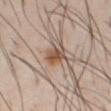{
  "image": {
    "source": "total-body photography crop",
    "field_of_view_mm": 15
  },
  "site": "abdomen",
  "patient": {
    "sex": "male",
    "age_approx": 40
  }
}